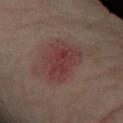<case>
<lesion_size>
  <long_diameter_mm_approx>5.0</long_diameter_mm_approx>
</lesion_size>
<image>
  <source>total-body photography crop</source>
  <field_of_view_mm>15</field_of_view_mm>
</image>
<site>left forearm</site>
<patient>
  <sex>female</sex>
  <age_approx>35</age_approx>
</patient>
</case>